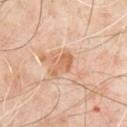| field | value |
|---|---|
| notes | no biopsy performed (imaged during a skin exam) |
| diameter | ~3 mm (longest diameter) |
| subject | male, aged 48 to 52 |
| imaging modality | ~15 mm crop, total-body skin-cancer survey |
| site | the chest |
| illumination | cross-polarized illumination |